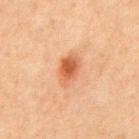Q: Was this lesion biopsied?
A: imaged on a skin check; not biopsied
Q: Where on the body is the lesion?
A: the abdomen
Q: How was this image acquired?
A: 15 mm crop, total-body photography
Q: Illumination type?
A: cross-polarized illumination
Q: Patient demographics?
A: male, aged 63 to 67
Q: How large is the lesion?
A: ~4 mm (longest diameter)